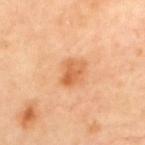The lesion was tiled from a total-body skin photograph and was not biopsied. Measured at roughly 3 mm in maximum diameter. This image is a 15 mm lesion crop taken from a total-body photograph. An algorithmic analysis of the crop reported a lesion area of about 6 mm² and a shape eccentricity near 0.75. The software also gave a border-irregularity rating of about 3/10, internal color variation of about 5 on a 0–10 scale, and radial color variation of about 1.5. It also reported a nevus-likeness score of about 75/100 and a lesion-detection confidence of about 100/100. A male patient in their mid- to late 60s. Captured under cross-polarized illumination. The lesion is located on the upper back.